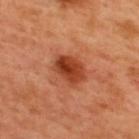notes — total-body-photography surveillance lesion; no biopsy
body site — the upper back
diameter — ≈4 mm
tile lighting — cross-polarized illumination
image source — ~15 mm tile from a whole-body skin photo
subject — male, in their 50s
TBP lesion metrics — a footprint of about 9 mm², an outline eccentricity of about 0.7 (0 = round, 1 = elongated), and two-axis asymmetry of about 0.15; a nevus-likeness score of about 95/100 and a lesion-detection confidence of about 100/100